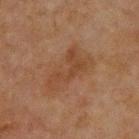Case summary:
– follow-up · no biopsy performed (imaged during a skin exam)
– size · about 5 mm
– automated metrics · an average lesion color of about L≈34 a*≈17 b*≈27 (CIELAB) and a lesion–skin lightness drop of about 6; border irregularity of about 8 on a 0–10 scale, a color-variation rating of about 2/10, and peripheral color asymmetry of about 0.5; a classifier nevus-likeness of about 0/100 and a lesion-detection confidence of about 100/100
– site · the chest
– subject · male, approximately 65 years of age
– lighting · cross-polarized illumination
– image · ~15 mm crop, total-body skin-cancer survey